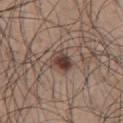| field | value |
|---|---|
| biopsy status | catalogued during a skin exam; not biopsied |
| lighting | white-light illumination |
| size | ~3 mm (longest diameter) |
| location | the upper back |
| subject | male, in their 50s |
| image-analysis metrics | an area of roughly 6 mm², an outline eccentricity of about 0.65 (0 = round, 1 = elongated), and a shape-asymmetry score of about 0.3 (0 = symmetric); an average lesion color of about L≈41 a*≈16 b*≈22 (CIELAB) and a lesion–skin lightness drop of about 12; border irregularity of about 2.5 on a 0–10 scale, a color-variation rating of about 7.5/10, and radial color variation of about 2.5 |
| image source | ~15 mm tile from a whole-body skin photo |